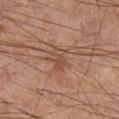<tbp_lesion>
<biopsy_status>not biopsied; imaged during a skin examination</biopsy_status>
<image>
  <source>total-body photography crop</source>
  <field_of_view_mm>15</field_of_view_mm>
</image>
<patient>
  <sex>male</sex>
  <age_approx>60</age_approx>
</patient>
<lesion_size>
  <long_diameter_mm_approx>3.5</long_diameter_mm_approx>
</lesion_size>
<site>left leg</site>
</tbp_lesion>